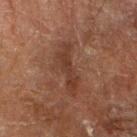Recorded during total-body skin imaging; not selected for excision or biopsy. The tile uses cross-polarized illumination. A roughly 15 mm field-of-view crop from a total-body skin photograph. Automated tile analysis of the lesion measured a mean CIELAB color near L≈29 a*≈18 b*≈23, about 7 CIELAB-L* units darker than the surrounding skin, and a normalized border contrast of about 7. The analysis additionally found a classifier nevus-likeness of about 0/100 and a lesion-detection confidence of about 75/100. Located on the right forearm. A male patient, roughly 65 years of age.